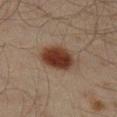Q: Is there a histopathology result?
A: total-body-photography surveillance lesion; no biopsy
Q: Patient demographics?
A: male, approximately 60 years of age
Q: Lesion location?
A: the leg
Q: What is the imaging modality?
A: ~15 mm tile from a whole-body skin photo